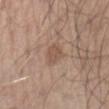Captured during whole-body skin photography for melanoma surveillance; the lesion was not biopsied.
The subject is a male aged 48–52.
Located on the left forearm.
A close-up tile cropped from a whole-body skin photograph, about 15 mm across.